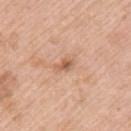Clinical impression: This lesion was catalogued during total-body skin photography and was not selected for biopsy. Acquisition and patient details: A region of skin cropped from a whole-body photographic capture, roughly 15 mm wide. Captured under white-light illumination. A male subject aged around 55. The lesion is on the left upper arm.